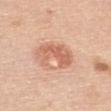follow-up: imaged on a skin check; not biopsied | patient: female, roughly 65 years of age | site: the upper back | imaging modality: total-body-photography crop, ~15 mm field of view.